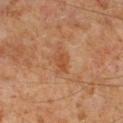notes = imaged on a skin check; not biopsied | image-analysis metrics = border irregularity of about 2.5 on a 0–10 scale and a peripheral color-asymmetry measure near 0.5 | lighting = cross-polarized | patient = male, approximately 60 years of age | lesion size = about 3 mm | site = the right lower leg | imaging modality = 15 mm crop, total-body photography.